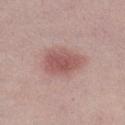| key | value |
|---|---|
| subject | female, about 20 years old |
| image source | ~15 mm crop, total-body skin-cancer survey |
| body site | the right lower leg |